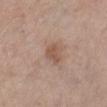Case summary:
- workup — imaged on a skin check; not biopsied
- illumination — white-light illumination
- image — 15 mm crop, total-body photography
- lesion diameter — ≈2.5 mm
- automated metrics — a lesion–skin lightness drop of about 8 and a lesion-to-skin contrast of about 6.5 (normalized; higher = more distinct); a border-irregularity rating of about 2.5/10, a within-lesion color-variation index near 1.5/10, and peripheral color asymmetry of about 0.5
- body site — the left lower leg
- patient — female, in their mid- to late 60s